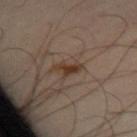biopsy status: imaged on a skin check; not biopsied
illumination: cross-polarized illumination
site: the abdomen
diameter: ~3.5 mm (longest diameter)
patient: male, approximately 45 years of age
image-analysis metrics: an area of roughly 4 mm², an eccentricity of roughly 0.9, and a symmetry-axis asymmetry near 0.5; a lesion color around L≈33 a*≈15 b*≈25 in CIELAB, roughly 8 lightness units darker than nearby skin, and a lesion-to-skin contrast of about 8.5 (normalized; higher = more distinct); border irregularity of about 5 on a 0–10 scale
imaging modality: ~15 mm tile from a whole-body skin photo